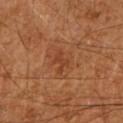Captured during whole-body skin photography for melanoma surveillance; the lesion was not biopsied. Cropped from a total-body skin-imaging series; the visible field is about 15 mm. The patient is a male about 60 years old. Located on the right upper arm. Longest diameter approximately 3 mm. The tile uses cross-polarized illumination.